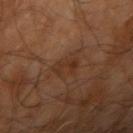workup: no biopsy performed (imaged during a skin exam)
image source: ~15 mm tile from a whole-body skin photo
subject: male, approximately 65 years of age
TBP lesion metrics: an average lesion color of about L≈29 a*≈19 b*≈27 (CIELAB), a lesion–skin lightness drop of about 5, and a normalized border contrast of about 6; border irregularity of about 5 on a 0–10 scale, internal color variation of about 1.5 on a 0–10 scale, and peripheral color asymmetry of about 0.5
size: ≈3 mm
location: the right upper arm